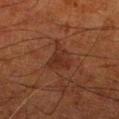* tile lighting — cross-polarized illumination
* lesion diameter — about 4 mm
* automated lesion analysis — an area of roughly 6.5 mm², an eccentricity of roughly 0.75, and a shape-asymmetry score of about 0.5 (0 = symmetric); an automated nevus-likeness rating near 10 out of 100 and a detector confidence of about 100 out of 100 that the crop contains a lesion
* subject — male, in their 80s
* image — ~15 mm crop, total-body skin-cancer survey
* body site — the right lower leg